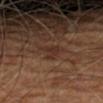follow-up = catalogued during a skin exam; not biopsied
automated lesion analysis = a nevus-likeness score of about 0/100 and lesion-presence confidence of about 100/100
illumination = cross-polarized
body site = the left forearm
acquisition = total-body-photography crop, ~15 mm field of view
patient = aged approximately 65